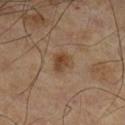Captured during whole-body skin photography for melanoma surveillance; the lesion was not biopsied.
A 15 mm close-up extracted from a 3D total-body photography capture.
From the left lower leg.
A male patient, roughly 60 years of age.